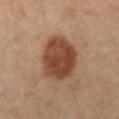This lesion was catalogued during total-body skin photography and was not selected for biopsy.
The patient is a female approximately 60 years of age.
Automated tile analysis of the lesion measured a mean CIELAB color near L≈38 a*≈19 b*≈28, a lesion–skin lightness drop of about 12, and a normalized border contrast of about 10. The analysis additionally found border irregularity of about 1.5 on a 0–10 scale, a within-lesion color-variation index near 3.5/10, and peripheral color asymmetry of about 1.5.
A close-up tile cropped from a whole-body skin photograph, about 15 mm across.
The recorded lesion diameter is about 5 mm.
From the right leg.
The tile uses cross-polarized illumination.